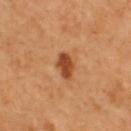site=the upper back; diameter=about 3 mm; patient=male, in their mid-40s; imaging modality=15 mm crop, total-body photography.